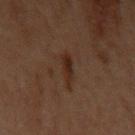A lesion tile, about 15 mm wide, cut from a 3D total-body photograph. On the left upper arm. The subject is a male approximately 60 years of age.